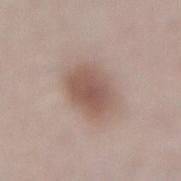Assessment: Part of a total-body skin-imaging series; this lesion was reviewed on a skin check and was not flagged for biopsy. Background: A 15 mm crop from a total-body photograph taken for skin-cancer surveillance. Automated tile analysis of the lesion measured border irregularity of about 1.5 on a 0–10 scale and radial color variation of about 1. And it measured a classifier nevus-likeness of about 95/100 and a detector confidence of about 100 out of 100 that the crop contains a lesion. A female subject aged around 80. This is a white-light tile. From the lower back. Longest diameter approximately 4.5 mm.